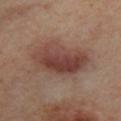| field | value |
|---|---|
| notes | catalogued during a skin exam; not biopsied |
| body site | the left lower leg |
| imaging modality | ~15 mm tile from a whole-body skin photo |
| patient | female, in their mid-50s |
| illumination | cross-polarized illumination |